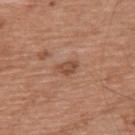<lesion>
  <image>
    <source>total-body photography crop</source>
    <field_of_view_mm>15</field_of_view_mm>
  </image>
  <site>back</site>
  <patient>
    <sex>male</sex>
    <age_approx>65</age_approx>
  </patient>
</lesion>